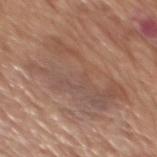Q: Patient demographics?
A: male, aged around 65
Q: What kind of image is this?
A: 15 mm crop, total-body photography
Q: What is the lesion's diameter?
A: ≈9.5 mm
Q: Where on the body is the lesion?
A: the mid back
Q: How was the tile lit?
A: white-light